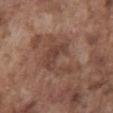biopsy status = no biopsy performed (imaged during a skin exam); location = the front of the torso; subject = male, aged 73–77; image source = 15 mm crop, total-body photography; lesion size = ≈5.5 mm.